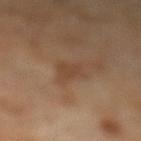Captured during whole-body skin photography for melanoma surveillance; the lesion was not biopsied.